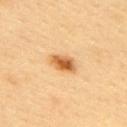No biopsy was performed on this lesion — it was imaged during a full skin examination and was not determined to be concerning.
Imaged with cross-polarized lighting.
This image is a 15 mm lesion crop taken from a total-body photograph.
The patient is a male roughly 40 years of age.
From the right upper arm.
The lesion's longest dimension is about 3.5 mm.
The total-body-photography lesion software estimated a border-irregularity index near 2/10 and a within-lesion color-variation index near 6/10. It also reported an automated nevus-likeness rating near 100 out of 100 and a lesion-detection confidence of about 100/100.